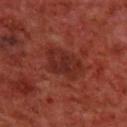The tile uses cross-polarized illumination. The subject is a male aged 68–72. A roughly 15 mm field-of-view crop from a total-body skin photograph. On the upper back.